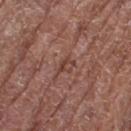Part of a total-body skin-imaging series; this lesion was reviewed on a skin check and was not flagged for biopsy. Approximately 2.5 mm at its widest. The lesion is on the left thigh. A female patient, aged approximately 80. Automated tile analysis of the lesion measured an area of roughly 3 mm², an eccentricity of roughly 0.8, and two-axis asymmetry of about 0.3. It also reported a nevus-likeness score of about 0/100 and lesion-presence confidence of about 55/100. Imaged with white-light lighting. Cropped from a whole-body photographic skin survey; the tile spans about 15 mm.